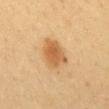This lesion was catalogued during total-body skin photography and was not selected for biopsy.
This is a cross-polarized tile.
Located on the mid back.
The patient is a female aged approximately 40.
This image is a 15 mm lesion crop taken from a total-body photograph.
The lesion's longest dimension is about 4.5 mm.